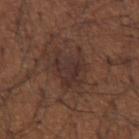Q: Automated lesion metrics?
A: an area of roughly 11 mm² and an outline eccentricity of about 0.45 (0 = round, 1 = elongated); a color-variation rating of about 2.5/10 and peripheral color asymmetry of about 1
Q: What lighting was used for the tile?
A: white-light illumination
Q: What kind of image is this?
A: 15 mm crop, total-body photography
Q: What is the anatomic site?
A: the arm
Q: How large is the lesion?
A: about 5 mm
Q: What are the patient's age and sex?
A: male, in their mid-60s